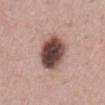body site = the abdomen; acquisition = 15 mm crop, total-body photography; subject = male, about 60 years old; histopathology = a dysplastic (Clark) nevus.